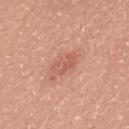Q: Is there a histopathology result?
A: no biopsy performed (imaged during a skin exam)
Q: Lesion location?
A: the upper back
Q: Who is the patient?
A: male, aged approximately 45
Q: How was the tile lit?
A: white-light
Q: What did automated image analysis measure?
A: an outline eccentricity of about 0.85 (0 = round, 1 = elongated) and a shape-asymmetry score of about 0.3 (0 = symmetric); a mean CIELAB color near L≈58 a*≈26 b*≈30, a lesion–skin lightness drop of about 8, and a normalized lesion–skin contrast near 5; border irregularity of about 3.5 on a 0–10 scale, internal color variation of about 3.5 on a 0–10 scale, and peripheral color asymmetry of about 1; a classifier nevus-likeness of about 5/100 and a detector confidence of about 100 out of 100 that the crop contains a lesion
Q: Lesion size?
A: ~3 mm (longest diameter)
Q: How was this image acquired?
A: ~15 mm tile from a whole-body skin photo